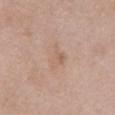follow-up: imaged on a skin check; not biopsied
image-analysis metrics: a lesion area of about 4.5 mm², an outline eccentricity of about 0.75 (0 = round, 1 = elongated), and a symmetry-axis asymmetry near 0.5; a border-irregularity index near 5/10, a color-variation rating of about 4/10, and a peripheral color-asymmetry measure near 1.5; a lesion-detection confidence of about 100/100
acquisition: total-body-photography crop, ~15 mm field of view
patient: female, aged 38 to 42
anatomic site: the chest
illumination: white-light
lesion diameter: ~3 mm (longest diameter)